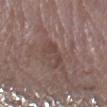biopsy status: imaged on a skin check; not biopsied
patient: female, aged approximately 70
image source: ~15 mm tile from a whole-body skin photo
TBP lesion metrics: a lesion area of about 4.5 mm² and a shape-asymmetry score of about 0.65 (0 = symmetric); border irregularity of about 8 on a 0–10 scale and a within-lesion color-variation index near 1/10
lighting: white-light
lesion size: ~3.5 mm (longest diameter)
anatomic site: the left forearm